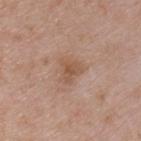Impression: The lesion was tiled from a total-body skin photograph and was not biopsied. Context: From the chest. Measured at roughly 3 mm in maximum diameter. A male subject aged 48 to 52. Automated tile analysis of the lesion measured border irregularity of about 3.5 on a 0–10 scale and a within-lesion color-variation index near 2.5/10. Imaged with white-light lighting. A region of skin cropped from a whole-body photographic capture, roughly 15 mm wide.